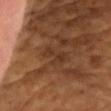Assessment:
This lesion was catalogued during total-body skin photography and was not selected for biopsy.
Acquisition and patient details:
A male subject aged 53–57. A 15 mm close-up tile from a total-body photography series done for melanoma screening. The lesion is on the chest.